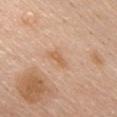{
  "biopsy_status": "not biopsied; imaged during a skin examination",
  "image": {
    "source": "total-body photography crop",
    "field_of_view_mm": 15
  },
  "site": "chest",
  "patient": {
    "sex": "male",
    "age_approx": 60
  }
}